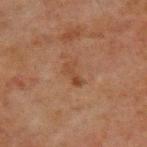Captured during whole-body skin photography for melanoma surveillance; the lesion was not biopsied. The lesion's longest dimension is about 3 mm. A lesion tile, about 15 mm wide, cut from a 3D total-body photograph. On the chest. This is a cross-polarized tile. A male patient approximately 65 years of age.